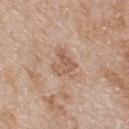Captured during whole-body skin photography for melanoma surveillance; the lesion was not biopsied.